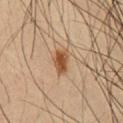The lesion was photographed on a routine skin check and not biopsied; there is no pathology result. Imaged with cross-polarized lighting. A region of skin cropped from a whole-body photographic capture, roughly 15 mm wide. A male subject aged approximately 35. The lesion is on the front of the torso. Approximately 3 mm at its widest.